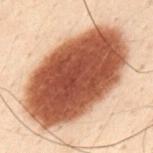Impression:
This lesion was catalogued during total-body skin photography and was not selected for biopsy.
Context:
A male patient, aged approximately 30. The recorded lesion diameter is about 12.5 mm. A 15 mm close-up extracted from a 3D total-body photography capture. The lesion is on the mid back.